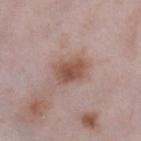The lesion was photographed on a routine skin check and not biopsied; there is no pathology result. Imaged with white-light lighting. Automated image analysis of the tile measured a footprint of about 9 mm², an outline eccentricity of about 0.65 (0 = round, 1 = elongated), and two-axis asymmetry of about 0.15. The analysis additionally found a within-lesion color-variation index near 3.5/10 and a peripheral color-asymmetry measure near 1. The patient is a female about 30 years old. On the leg. Approximately 3.5 mm at its widest. A region of skin cropped from a whole-body photographic capture, roughly 15 mm wide.